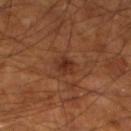- follow-up: imaged on a skin check; not biopsied
- lesion diameter: ~2.5 mm (longest diameter)
- location: the left lower leg
- subject: male, aged approximately 60
- acquisition: ~15 mm crop, total-body skin-cancer survey
- illumination: cross-polarized illumination